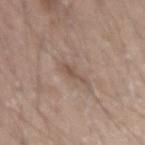patient:
  sex: male
  age_approx: 20
automated_metrics:
  cielab_L: 51
  cielab_a: 15
  cielab_b: 25
  vs_skin_darker_L: 8.0
  vs_skin_contrast_norm: 6.0
  color_variation_0_10: 0.5
  nevus_likeness_0_100: 0
lighting: white-light
lesion_size:
  long_diameter_mm_approx: 3.0
site: right forearm
image:
  source: total-body photography crop
  field_of_view_mm: 15